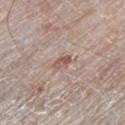<record>
  <biopsy_status>not biopsied; imaged during a skin examination</biopsy_status>
  <site>left lower leg</site>
  <lighting>white-light</lighting>
  <automated_metrics>
    <nevus_likeness_0_100>0</nevus_likeness_0_100>
    <lesion_detection_confidence_0_100>100</lesion_detection_confidence_0_100>
  </automated_metrics>
  <image>
    <source>total-body photography crop</source>
    <field_of_view_mm>15</field_of_view_mm>
  </image>
  <patient>
    <sex>male</sex>
    <age_approx>80</age_approx>
  </patient>
  <lesion_size>
    <long_diameter_mm_approx>2.5</long_diameter_mm_approx>
  </lesion_size>
</record>